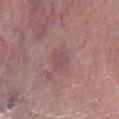Q: Was a biopsy performed?
A: imaged on a skin check; not biopsied
Q: Where on the body is the lesion?
A: the right lower leg
Q: What kind of image is this?
A: ~15 mm tile from a whole-body skin photo
Q: Patient demographics?
A: female, aged around 65